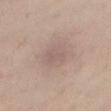Captured during whole-body skin photography for melanoma surveillance; the lesion was not biopsied. Measured at roughly 3 mm in maximum diameter. The lesion is located on the right thigh. Cropped from a whole-body photographic skin survey; the tile spans about 15 mm. Automated tile analysis of the lesion measured a color-variation rating of about 2/10 and radial color variation of about 0.5. The analysis additionally found an automated nevus-likeness rating near 0 out of 100. A female subject in their 40s.